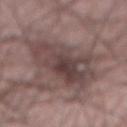The lesion is located on the front of the torso. This image is a 15 mm lesion crop taken from a total-body photograph. A male subject approximately 60 years of age.